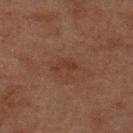The lesion was tiled from a total-body skin photograph and was not biopsied.